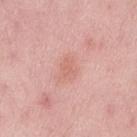The lesion was photographed on a routine skin check and not biopsied; there is no pathology result.
The tile uses white-light illumination.
The lesion is on the lower back.
A close-up tile cropped from a whole-body skin photograph, about 15 mm across.
The recorded lesion diameter is about 3 mm.
A female patient aged 48 to 52.